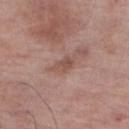Assessment:
Captured during whole-body skin photography for melanoma surveillance; the lesion was not biopsied.
Context:
This is a white-light tile. The lesion is located on the right lower leg. The lesion's longest dimension is about 3 mm. A male subject, approximately 70 years of age. This image is a 15 mm lesion crop taken from a total-body photograph. The total-body-photography lesion software estimated border irregularity of about 3.5 on a 0–10 scale, a within-lesion color-variation index near 1/10, and a peripheral color-asymmetry measure near 0.5. The software also gave a classifier nevus-likeness of about 0/100 and lesion-presence confidence of about 100/100.